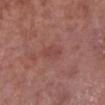follow-up: no biopsy performed (imaged during a skin exam); image: 15 mm crop, total-body photography; image-analysis metrics: border irregularity of about 1.5 on a 0–10 scale, internal color variation of about 1.5 on a 0–10 scale, and a peripheral color-asymmetry measure near 0.5; lesion diameter: ≈3 mm; body site: the right lower leg; illumination: white-light illumination; patient: male, aged approximately 55.